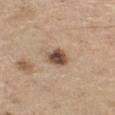The lesion was tiled from a total-body skin photograph and was not biopsied. A close-up tile cropped from a whole-body skin photograph, about 15 mm across. About 2.5 mm across. The total-body-photography lesion software estimated an area of roughly 5.5 mm², a shape eccentricity near 0.3, and a shape-asymmetry score of about 0.15 (0 = symmetric). The software also gave roughly 17 lightness units darker than nearby skin. The patient is a male in their 70s. The lesion is on the leg.